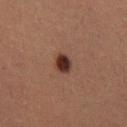The lesion was photographed on a routine skin check and not biopsied; there is no pathology result. Located on the right thigh. A female patient, about 40 years old. A 15 mm close-up tile from a total-body photography series done for melanoma screening.